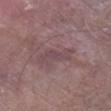workup: catalogued during a skin exam; not biopsied
anatomic site: the leg
subject: male, aged 78–82
image source: ~15 mm tile from a whole-body skin photo